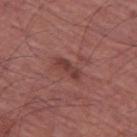  biopsy_status: not biopsied; imaged during a skin examination
  patient:
    sex: male
    age_approx: 65
  site: left thigh
  image:
    source: total-body photography crop
    field_of_view_mm: 15